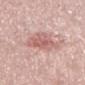<case>
  <biopsy_status>not biopsied; imaged during a skin examination</biopsy_status>
  <patient>
    <sex>female</sex>
    <age_approx>40</age_approx>
  </patient>
  <site>left thigh</site>
  <lighting>white-light</lighting>
  <image>
    <source>total-body photography crop</source>
    <field_of_view_mm>15</field_of_view_mm>
  </image>
</case>